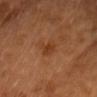Part of a total-body skin-imaging series; this lesion was reviewed on a skin check and was not flagged for biopsy.
On the head or neck.
A male subject, approximately 40 years of age.
Cropped from a whole-body photographic skin survey; the tile spans about 15 mm.